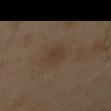The lesion was photographed on a routine skin check and not biopsied; there is no pathology result. Located on the right forearm. Cropped from a total-body skin-imaging series; the visible field is about 15 mm. A male subject, in their mid-40s.